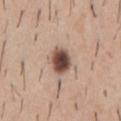Assessment:
Part of a total-body skin-imaging series; this lesion was reviewed on a skin check and was not flagged for biopsy.
Acquisition and patient details:
Imaged with white-light lighting. The lesion's longest dimension is about 3 mm. The patient is a male aged 38–42. Cropped from a whole-body photographic skin survey; the tile spans about 15 mm. The lesion is on the chest.